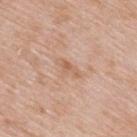Q: Was a biopsy performed?
A: no biopsy performed (imaged during a skin exam)
Q: What kind of image is this?
A: ~15 mm tile from a whole-body skin photo
Q: Lesion location?
A: the upper back
Q: What is the lesion's diameter?
A: ~3 mm (longest diameter)
Q: Who is the patient?
A: male, aged approximately 65